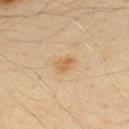No biopsy was performed on this lesion — it was imaged during a full skin examination and was not determined to be concerning.
Located on the upper back.
The lesion-visualizer software estimated a shape eccentricity near 0.75. The analysis additionally found an average lesion color of about L≈56 a*≈15 b*≈36 (CIELAB), a lesion–skin lightness drop of about 7, and a normalized border contrast of about 6.5. The analysis additionally found a lesion-detection confidence of about 100/100.
This is a cross-polarized tile.
A 15 mm crop from a total-body photograph taken for skin-cancer surveillance.
A male patient in their mid- to late 30s.